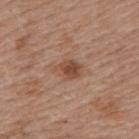No biopsy was performed on this lesion — it was imaged during a full skin examination and was not determined to be concerning.
On the upper back.
A female patient, about 60 years old.
Cropped from a whole-body photographic skin survey; the tile spans about 15 mm.
Longest diameter approximately 3.5 mm.
The lesion-visualizer software estimated roughly 10 lightness units darker than nearby skin. And it measured a detector confidence of about 100 out of 100 that the crop contains a lesion.